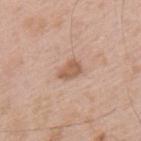Clinical impression: Part of a total-body skin-imaging series; this lesion was reviewed on a skin check and was not flagged for biopsy. Acquisition and patient details: Located on the upper back. A 15 mm close-up tile from a total-body photography series done for melanoma screening. A male patient, approximately 50 years of age. The recorded lesion diameter is about 3 mm.